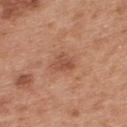Notes:
– notes — catalogued during a skin exam; not biopsied
– imaging modality — 15 mm crop, total-body photography
– lesion diameter — ~2.5 mm (longest diameter)
– lighting — white-light
– body site — the upper back
– subject — male, in their 30s
– automated lesion analysis — a mean CIELAB color near L≈51 a*≈25 b*≈32 and a normalized lesion–skin contrast near 6